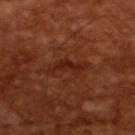Q: Is there a histopathology result?
A: catalogued during a skin exam; not biopsied
Q: Who is the patient?
A: male, in their mid-60s
Q: Automated lesion metrics?
A: a mean CIELAB color near L≈25 a*≈25 b*≈28
Q: How was this image acquired?
A: ~15 mm crop, total-body skin-cancer survey
Q: What lighting was used for the tile?
A: cross-polarized illumination
Q: How large is the lesion?
A: ≈4.5 mm